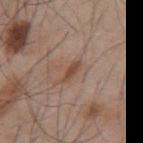No biopsy was performed on this lesion — it was imaged during a full skin examination and was not determined to be concerning. Captured under white-light illumination. About 3 mm across. Cropped from a whole-body photographic skin survey; the tile spans about 15 mm. The total-body-photography lesion software estimated an outline eccentricity of about 0.9 (0 = round, 1 = elongated) and two-axis asymmetry of about 0.3. And it measured roughly 9 lightness units darker than nearby skin. The analysis additionally found border irregularity of about 3 on a 0–10 scale. And it measured a classifier nevus-likeness of about 0/100 and a detector confidence of about 100 out of 100 that the crop contains a lesion. On the mid back. The subject is a male aged around 55.